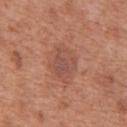notes = total-body-photography surveillance lesion; no biopsy | lesion diameter = about 4 mm | image source = total-body-photography crop, ~15 mm field of view | subject = male, about 65 years old | anatomic site = the chest.